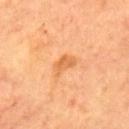<record>
<biopsy_status>not biopsied; imaged during a skin examination</biopsy_status>
<lighting>cross-polarized</lighting>
<image>
  <source>total-body photography crop</source>
  <field_of_view_mm>15</field_of_view_mm>
</image>
<site>upper back</site>
<patient>
  <sex>male</sex>
  <age_approx>70</age_approx>
</patient>
<lesion_size>
  <long_diameter_mm_approx>3.0</long_diameter_mm_approx>
</lesion_size>
<automated_metrics>
  <cielab_L>63</cielab_L>
  <cielab_a>27</cielab_a>
  <cielab_b>45</cielab_b>
  <vs_skin_darker_L>9.0</vs_skin_darker_L>
  <vs_skin_contrast_norm>6.0</vs_skin_contrast_norm>
</automated_metrics>
</record>